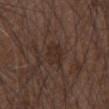This lesion was catalogued during total-body skin photography and was not selected for biopsy. Cropped from a whole-body photographic skin survey; the tile spans about 15 mm. Automated tile analysis of the lesion measured an automated nevus-likeness rating near 5 out of 100. This is a white-light tile. Located on the right forearm. Approximately 2.5 mm at its widest. A male patient roughly 50 years of age.